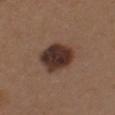| field | value |
|---|---|
| notes | total-body-photography surveillance lesion; no biopsy |
| subject | female, aged approximately 40 |
| illumination | white-light |
| acquisition | total-body-photography crop, ~15 mm field of view |
| location | the left upper arm |
| TBP lesion metrics | a lesion area of about 15 mm², a shape eccentricity near 0.55, and a shape-asymmetry score of about 0.15 (0 = symmetric); an average lesion color of about L≈33 a*≈17 b*≈22 (CIELAB), roughly 15 lightness units darker than nearby skin, and a lesion-to-skin contrast of about 13 (normalized; higher = more distinct) |
| lesion diameter | ≈4.5 mm |